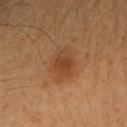A close-up tile cropped from a whole-body skin photograph, about 15 mm across. The subject is a female aged 48–52. On the left forearm.